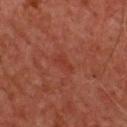Assessment:
Imaged during a routine full-body skin examination; the lesion was not biopsied and no histopathology is available.
Background:
Captured under cross-polarized illumination. A 15 mm close-up tile from a total-body photography series done for melanoma screening. The lesion is located on the chest. A male patient, approximately 60 years of age.